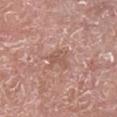Part of a total-body skin-imaging series; this lesion was reviewed on a skin check and was not flagged for biopsy. Cropped from a whole-body photographic skin survey; the tile spans about 15 mm. A male subject in their mid-70s. On the right lower leg.